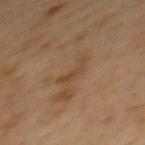{"biopsy_status": "not biopsied; imaged during a skin examination", "site": "mid back", "image": {"source": "total-body photography crop", "field_of_view_mm": 15}, "lighting": "cross-polarized", "patient": {"sex": "male", "age_approx": 50}, "automated_metrics": {"cielab_L": 44, "cielab_a": 17, "cielab_b": 33, "vs_skin_contrast_norm": 5.5, "border_irregularity_0_10": 8.0, "color_variation_0_10": 0.0, "peripheral_color_asymmetry": 0.0, "lesion_detection_confidence_0_100": 100}, "lesion_size": {"long_diameter_mm_approx": 3.5}}